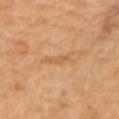Acquisition and patient details: This is a cross-polarized tile. On the chest. The patient is a female aged around 70. The lesion-visualizer software estimated a lesion–skin lightness drop of about 7 and a normalized lesion–skin contrast near 5. The analysis additionally found a border-irregularity index near 4.5/10, a color-variation rating of about 0/10, and radial color variation of about 0. This image is a 15 mm lesion crop taken from a total-body photograph. Approximately 3 mm at its widest.